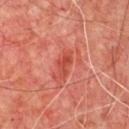Q: Was this lesion biopsied?
A: total-body-photography surveillance lesion; no biopsy
Q: What did automated image analysis measure?
A: roughly 9 lightness units darker than nearby skin and a normalized border contrast of about 6.5; a classifier nevus-likeness of about 5/100 and lesion-presence confidence of about 100/100
Q: What is the lesion's diameter?
A: ≈3 mm
Q: What kind of image is this?
A: ~15 mm tile from a whole-body skin photo
Q: Where on the body is the lesion?
A: the chest
Q: What are the patient's age and sex?
A: male, approximately 65 years of age
Q: Illumination type?
A: cross-polarized